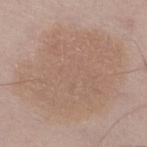follow-up — no biopsy performed (imaged during a skin exam); subject — male, aged around 60; anatomic site — the right thigh; acquisition — 15 mm crop, total-body photography.